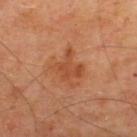Clinical impression: The lesion was photographed on a routine skin check and not biopsied; there is no pathology result. Acquisition and patient details: This image is a 15 mm lesion crop taken from a total-body photograph. The lesion is located on the upper back. The total-body-photography lesion software estimated a within-lesion color-variation index near 3/10. Captured under cross-polarized illumination. The recorded lesion diameter is about 4 mm. A male patient aged approximately 65.